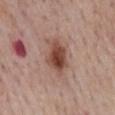Clinical impression:
Captured during whole-body skin photography for melanoma surveillance; the lesion was not biopsied.
Background:
A male patient in their mid-70s. Located on the mid back. The tile uses white-light illumination. A 15 mm close-up extracted from a 3D total-body photography capture. The lesion-visualizer software estimated an area of roughly 10 mm² and an eccentricity of roughly 0.75.